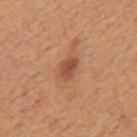Recorded during total-body skin imaging; not selected for excision or biopsy.